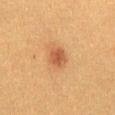| key | value |
|---|---|
| biopsy status | total-body-photography surveillance lesion; no biopsy |
| imaging modality | 15 mm crop, total-body photography |
| patient | female, roughly 40 years of age |
| automated metrics | a mean CIELAB color near L≈45 a*≈21 b*≈33, about 9 CIELAB-L* units darker than the surrounding skin, and a normalized lesion–skin contrast near 7; a border-irregularity rating of about 1.5/10, internal color variation of about 3.5 on a 0–10 scale, and radial color variation of about 1; a lesion-detection confidence of about 100/100 |
| tile lighting | cross-polarized illumination |
| lesion diameter | about 2.5 mm |
| site | the abdomen |